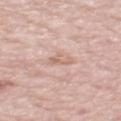notes: total-body-photography surveillance lesion; no biopsy
lesion diameter: about 2.5 mm
body site: the back
patient: male, aged around 70
tile lighting: white-light illumination
image source: total-body-photography crop, ~15 mm field of view